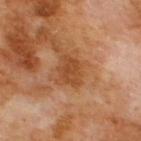workup: catalogued during a skin exam; not biopsied | body site: the back | acquisition: ~15 mm crop, total-body skin-cancer survey | subject: male, aged 68 to 72.